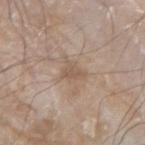– TBP lesion metrics: a shape-asymmetry score of about 0.35 (0 = symmetric); an average lesion color of about L≈55 a*≈15 b*≈27 (CIELAB), roughly 8 lightness units darker than nearby skin, and a lesion-to-skin contrast of about 5.5 (normalized; higher = more distinct); border irregularity of about 3.5 on a 0–10 scale, a within-lesion color-variation index near 1/10, and peripheral color asymmetry of about 0.5
– location: the arm
– image: 15 mm crop, total-body photography
– illumination: white-light
– size: ~2.5 mm (longest diameter)
– subject: male, in their 80s